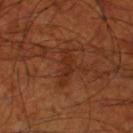Findings:
- biopsy status · no biopsy performed (imaged during a skin exam)
- imaging modality · 15 mm crop, total-body photography
- tile lighting · cross-polarized illumination
- diameter · ~4.5 mm (longest diameter)
- location · the leg
- patient · male, aged 68 to 72